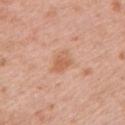<record>
  <biopsy_status>not biopsied; imaged during a skin examination</biopsy_status>
  <site>left upper arm</site>
  <lesion_size>
    <long_diameter_mm_approx>2.5</long_diameter_mm_approx>
  </lesion_size>
  <automated_metrics>
    <vs_skin_darker_L>8.0</vs_skin_darker_L>
    <border_irregularity_0_10>2.0</border_irregularity_0_10>
    <color_variation_0_10>3.0</color_variation_0_10>
    <peripheral_color_asymmetry>1.0</peripheral_color_asymmetry>
  </automated_metrics>
  <image>
    <source>total-body photography crop</source>
    <field_of_view_mm>15</field_of_view_mm>
  </image>
  <lighting>white-light</lighting>
  <patient>
    <sex>female</sex>
    <age_approx>40</age_approx>
  </patient>
</record>